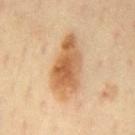workup — catalogued during a skin exam; not biopsied | subject — male, aged 63 to 67 | image — ~15 mm tile from a whole-body skin photo | automated metrics — a lesion–skin lightness drop of about 13 and a lesion-to-skin contrast of about 8.5 (normalized; higher = more distinct); a border-irregularity rating of about 3.5/10, a within-lesion color-variation index near 5.5/10, and radial color variation of about 1.5; a classifier nevus-likeness of about 90/100 and a lesion-detection confidence of about 100/100 | location — the front of the torso.